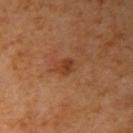workup — catalogued during a skin exam; not biopsied
site — the right upper arm
diameter — ≈2.5 mm
illumination — cross-polarized illumination
subject — male, in their 60s
acquisition — ~15 mm tile from a whole-body skin photo